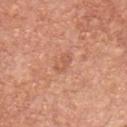{"biopsy_status": "not biopsied; imaged during a skin examination", "image": {"source": "total-body photography crop", "field_of_view_mm": 15}, "site": "chest", "lesion_size": {"long_diameter_mm_approx": 2.5}, "lighting": "white-light", "patient": {"sex": "male", "age_approx": 65}}